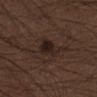notes: catalogued during a skin exam; not biopsied | patient: male, aged around 50 | location: the left lower leg | acquisition: 15 mm crop, total-body photography | TBP lesion metrics: a footprint of about 6.5 mm², a shape eccentricity near 0.75, and two-axis asymmetry of about 0.4; a lesion color around L≈21 a*≈12 b*≈16 in CIELAB and about 8 CIELAB-L* units darker than the surrounding skin; a classifier nevus-likeness of about 45/100 and a lesion-detection confidence of about 100/100.